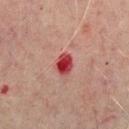image: ~15 mm tile from a whole-body skin photo | automated metrics: an area of roughly 5 mm² and two-axis asymmetry of about 0.25; a lesion color around L≈36 a*≈34 b*≈23 in CIELAB, roughly 14 lightness units darker than nearby skin, and a lesion-to-skin contrast of about 11.5 (normalized; higher = more distinct); a classifier nevus-likeness of about 0/100 and a detector confidence of about 100 out of 100 that the crop contains a lesion | lesion size: ≈3 mm | body site: the mid back | subject: male, aged approximately 70 | illumination: cross-polarized.